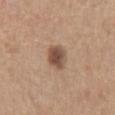- notes · no biopsy performed (imaged during a skin exam)
- subject · male, in their mid-60s
- image source · ~15 mm tile from a whole-body skin photo
- size · about 2.5 mm
- body site · the mid back
- automated lesion analysis · a lesion area of about 6 mm², a shape eccentricity near 0.45, and two-axis asymmetry of about 0.25; roughly 13 lightness units darker than nearby skin and a lesion-to-skin contrast of about 9.5 (normalized; higher = more distinct); a border-irregularity rating of about 2/10, a within-lesion color-variation index near 4.5/10, and radial color variation of about 1.5; a classifier nevus-likeness of about 90/100 and a detector confidence of about 100 out of 100 that the crop contains a lesion